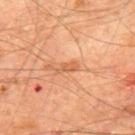workup: no biopsy performed (imaged during a skin exam)
TBP lesion metrics: a border-irregularity rating of about 4/10, a within-lesion color-variation index near 0/10, and peripheral color asymmetry of about 0; a classifier nevus-likeness of about 0/100
image source: total-body-photography crop, ~15 mm field of view
subject: male, aged approximately 65
size: ~2.5 mm (longest diameter)
location: the back